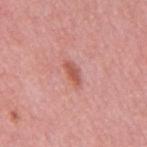The lesion was tiled from a total-body skin photograph and was not biopsied.
This is a white-light tile.
A male subject roughly 50 years of age.
This image is a 15 mm lesion crop taken from a total-body photograph.
Longest diameter approximately 3 mm.
An algorithmic analysis of the crop reported a lesion color around L≈56 a*≈29 b*≈29 in CIELAB, roughly 10 lightness units darker than nearby skin, and a lesion-to-skin contrast of about 7.5 (normalized; higher = more distinct). The analysis additionally found an automated nevus-likeness rating near 40 out of 100 and a lesion-detection confidence of about 100/100.
Located on the mid back.